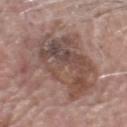Notes:
– notes — imaged on a skin check; not biopsied